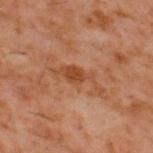  patient:
    sex: male
    age_approx: 60
  automated_metrics:
    nevus_likeness_0_100: 0
    lesion_detection_confidence_0_100: 100
  site: back
  image:
    source: total-body photography crop
    field_of_view_mm: 15
  lighting: cross-polarized
  lesion_size:
    long_diameter_mm_approx: 6.0